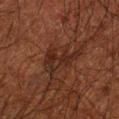workup = total-body-photography surveillance lesion; no biopsy | acquisition = ~15 mm tile from a whole-body skin photo | site = the right forearm | patient = male, approximately 50 years of age.